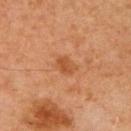  biopsy_status: not biopsied; imaged during a skin examination
  lighting: cross-polarized
  site: right upper arm
  patient:
    sex: male
    age_approx: 60
  image:
    source: total-body photography crop
    field_of_view_mm: 15
  lesion_size:
    long_diameter_mm_approx: 2.5